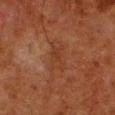This lesion was catalogued during total-body skin photography and was not selected for biopsy.
Approximately 3 mm at its widest.
A male subject aged 78 to 82.
The lesion is on the left lower leg.
Cropped from a total-body skin-imaging series; the visible field is about 15 mm.
Automated image analysis of the tile measured a mean CIELAB color near L≈29 a*≈20 b*≈27, a lesion–skin lightness drop of about 4, and a normalized border contrast of about 5. The software also gave a nevus-likeness score of about 0/100.
The tile uses cross-polarized illumination.